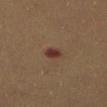<lesion>
  <biopsy_status>not biopsied; imaged during a skin examination</biopsy_status>
  <patient>
    <sex>male</sex>
    <age_approx>60</age_approx>
  </patient>
  <image>
    <source>total-body photography crop</source>
    <field_of_view_mm>15</field_of_view_mm>
  </image>
  <site>abdomen</site>
  <lighting>cross-polarized</lighting>
</lesion>